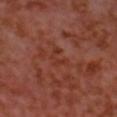  biopsy_status: not biopsied; imaged during a skin examination
  patient:
    sex: male
    age_approx: 55
  lighting: cross-polarized
  site: head or neck
  lesion_size:
    long_diameter_mm_approx: 3.0
  image:
    source: total-body photography crop
    field_of_view_mm: 15